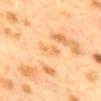subject — female, aged 38–42 | image source — ~15 mm tile from a whole-body skin photo | automated lesion analysis — border irregularity of about 3 on a 0–10 scale, a within-lesion color-variation index near 1.5/10, and peripheral color asymmetry of about 0.5; an automated nevus-likeness rating near 0 out of 100 and a detector confidence of about 100 out of 100 that the crop contains a lesion | anatomic site — the back.